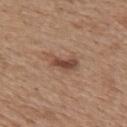  biopsy_status: not biopsied; imaged during a skin examination
  site: upper back
  lesion_size:
    long_diameter_mm_approx: 4.0
  lighting: white-light
  image:
    source: total-body photography crop
    field_of_view_mm: 15
  patient:
    sex: female
    age_approx: 45
  automated_metrics:
    area_mm2_approx: 6.0
    eccentricity: 0.9
    shape_asymmetry: 0.35
    border_irregularity_0_10: 4.0
    color_variation_0_10: 4.0
    peripheral_color_asymmetry: 1.0